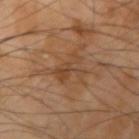Case summary:
* size — ≈4 mm
* subject — male, roughly 65 years of age
* lighting — cross-polarized
* site — the right upper arm
* imaging modality — ~15 mm tile from a whole-body skin photo